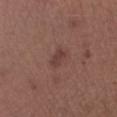Impression: The lesion was tiled from a total-body skin photograph and was not biopsied. Background: The lesion is located on the left lower leg. The lesion's longest dimension is about 2.5 mm. A lesion tile, about 15 mm wide, cut from a 3D total-body photograph. Automated image analysis of the tile measured a within-lesion color-variation index near 2.5/10 and peripheral color asymmetry of about 1. And it measured an automated nevus-likeness rating near 15 out of 100 and a lesion-detection confidence of about 100/100. The subject is a female aged 53 to 57. Imaged with white-light lighting.